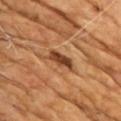| key | value |
|---|---|
| workup | imaged on a skin check; not biopsied |
| imaging modality | ~15 mm crop, total-body skin-cancer survey |
| lesion size | ~4 mm (longest diameter) |
| body site | the front of the torso |
| tile lighting | cross-polarized |
| subject | male, aged around 70 |
| TBP lesion metrics | about 13 CIELAB-L* units darker than the surrounding skin and a lesion-to-skin contrast of about 10 (normalized; higher = more distinct); a border-irregularity index near 4.5/10, internal color variation of about 3.5 on a 0–10 scale, and a peripheral color-asymmetry measure near 1.5; a nevus-likeness score of about 0/100 |